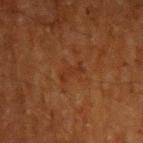- follow-up · catalogued during a skin exam; not biopsied
- image source · ~15 mm tile from a whole-body skin photo
- diameter · ≈3 mm
- subject · male, aged around 60
- anatomic site · the left upper arm
- illumination · cross-polarized illumination